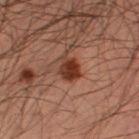  biopsy_status: not biopsied; imaged during a skin examination
  automated_metrics:
    cielab_L: 32
    cielab_a: 23
    cielab_b: 27
    border_irregularity_0_10: 2.5
    color_variation_0_10: 4.0
    peripheral_color_asymmetry: 1.5
    nevus_likeness_0_100: 95
    lesion_detection_confidence_0_100: 100
  image:
    source: total-body photography crop
    field_of_view_mm: 15
  site: left lower leg
  patient:
    sex: male
    age_approx: 35
  lighting: cross-polarized